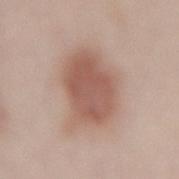Impression:
No biopsy was performed on this lesion — it was imaged during a full skin examination and was not determined to be concerning.
Acquisition and patient details:
The patient is a female in their mid- to late 60s. A lesion tile, about 15 mm wide, cut from a 3D total-body photograph. About 7 mm across. The lesion is on the mid back. This is a white-light tile. Automated image analysis of the tile measured border irregularity of about 2.5 on a 0–10 scale, a color-variation rating of about 3.5/10, and radial color variation of about 1. The software also gave a lesion-detection confidence of about 100/100.